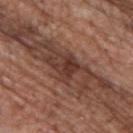Acquisition and patient details:
A lesion tile, about 15 mm wide, cut from a 3D total-body photograph. A male subject, in their 70s. The lesion is on the upper back. The lesion's longest dimension is about 6 mm. Captured under white-light illumination.